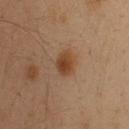Findings:
* follow-up · catalogued during a skin exam; not biopsied
* image-analysis metrics · an eccentricity of roughly 0.6 and two-axis asymmetry of about 0.25; a border-irregularity rating of about 2/10 and peripheral color asymmetry of about 1
* lesion diameter · ≈2.5 mm
* tile lighting · cross-polarized illumination
* body site · the back
* imaging modality · ~15 mm tile from a whole-body skin photo
* subject · male, aged 33 to 37